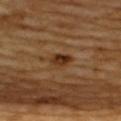| feature | finding |
|---|---|
| notes | no biopsy performed (imaged during a skin exam) |
| image | ~15 mm crop, total-body skin-cancer survey |
| patient | male, aged 58 to 62 |
| lighting | cross-polarized |
| TBP lesion metrics | an area of roughly 4.5 mm², a shape eccentricity near 0.6, and a symmetry-axis asymmetry near 0.2; border irregularity of about 2 on a 0–10 scale, a color-variation rating of about 5/10, and a peripheral color-asymmetry measure near 2 |
| location | the upper back |